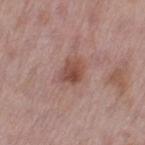Captured during whole-body skin photography for melanoma surveillance; the lesion was not biopsied. Approximately 2.5 mm at its widest. Located on the leg. A female subject, in their 50s. A 15 mm close-up tile from a total-body photography series done for melanoma screening. The tile uses white-light illumination.